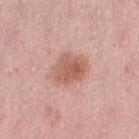Case summary:
* follow-up: imaged on a skin check; not biopsied
* imaging modality: ~15 mm tile from a whole-body skin photo
* lighting: white-light illumination
* location: the leg
* patient: female, aged approximately 40
* diameter: ~4 mm (longest diameter)